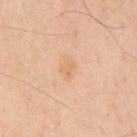{"biopsy_status": "not biopsied; imaged during a skin examination", "image": {"source": "total-body photography crop", "field_of_view_mm": 15}, "site": "mid back", "patient": {"sex": "male", "age_approx": 45}}